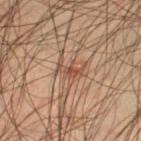* biopsy status — no biopsy performed (imaged during a skin exam)
* tile lighting — cross-polarized
* size — ≈3 mm
* location — the left thigh
* image source — 15 mm crop, total-body photography
* patient — male, in their mid- to late 40s